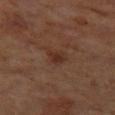| feature | finding |
|---|---|
| notes | catalogued during a skin exam; not biopsied |
| subject | male, aged around 60 |
| body site | the leg |
| acquisition | ~15 mm tile from a whole-body skin photo |
| tile lighting | cross-polarized |
| lesion diameter | ≈2.5 mm |